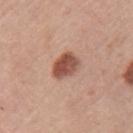Background: The lesion's longest dimension is about 3.5 mm. Captured under white-light illumination. The patient is a female roughly 60 years of age. The lesion is on the left upper arm. An algorithmic analysis of the crop reported a footprint of about 7.5 mm², a shape eccentricity near 0.55, and two-axis asymmetry of about 0.2. A 15 mm close-up tile from a total-body photography series done for melanoma screening.